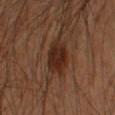Context:
This is a cross-polarized tile. The recorded lesion diameter is about 4 mm. On the left forearm. A 15 mm crop from a total-body photograph taken for skin-cancer surveillance. A male subject, approximately 50 years of age.